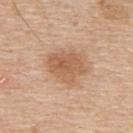Q: Is there a histopathology result?
A: total-body-photography surveillance lesion; no biopsy
Q: What is the anatomic site?
A: the upper back
Q: What kind of image is this?
A: ~15 mm crop, total-body skin-cancer survey
Q: What lighting was used for the tile?
A: white-light
Q: Who is the patient?
A: male, aged around 60